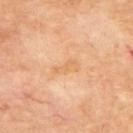Q: Was this lesion biopsied?
A: total-body-photography surveillance lesion; no biopsy
Q: What did automated image analysis measure?
A: an average lesion color of about L≈66 a*≈22 b*≈41 (CIELAB) and a lesion–skin lightness drop of about 6; border irregularity of about 6.5 on a 0–10 scale and a within-lesion color-variation index near 0/10; a classifier nevus-likeness of about 0/100
Q: What lighting was used for the tile?
A: cross-polarized illumination
Q: What kind of image is this?
A: 15 mm crop, total-body photography
Q: Patient demographics?
A: male, aged 68 to 72
Q: Lesion size?
A: ≈3 mm
Q: Where on the body is the lesion?
A: the upper back